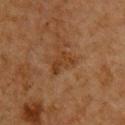biopsy status: no biopsy performed (imaged during a skin exam) | image source: 15 mm crop, total-body photography | anatomic site: the front of the torso | image-analysis metrics: a lesion color around L≈32 a*≈18 b*≈30 in CIELAB, a lesion–skin lightness drop of about 6, and a lesion-to-skin contrast of about 6.5 (normalized; higher = more distinct) | patient: male, roughly 60 years of age.